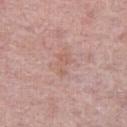Part of a total-body skin-imaging series; this lesion was reviewed on a skin check and was not flagged for biopsy.
The lesion is on the right thigh.
Measured at roughly 3 mm in maximum diameter.
A female patient approximately 65 years of age.
Captured under white-light illumination.
A 15 mm close-up tile from a total-body photography series done for melanoma screening.